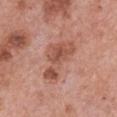The lesion was tiled from a total-body skin photograph and was not biopsied.
A female patient, aged around 60.
Approximately 6 mm at its widest.
Automated tile analysis of the lesion measured a lesion area of about 14 mm², a shape eccentricity near 0.85, and a shape-asymmetry score of about 0.5 (0 = symmetric). The analysis additionally found a border-irregularity rating of about 5.5/10, a within-lesion color-variation index near 5.5/10, and peripheral color asymmetry of about 2.
This is a white-light tile.
From the chest.
A 15 mm crop from a total-body photograph taken for skin-cancer surveillance.